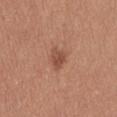Clinical impression: The lesion was tiled from a total-body skin photograph and was not biopsied. Background: Located on the lower back. Measured at roughly 2.5 mm in maximum diameter. The total-body-photography lesion software estimated a footprint of about 3.5 mm², an eccentricity of roughly 0.65, and a symmetry-axis asymmetry near 0.35. It also reported roughly 10 lightness units darker than nearby skin and a normalized lesion–skin contrast near 7. The analysis additionally found a border-irregularity index near 3/10 and a within-lesion color-variation index near 2/10. And it measured an automated nevus-likeness rating near 65 out of 100. Cropped from a total-body skin-imaging series; the visible field is about 15 mm. The tile uses white-light illumination. The subject is a female aged 38–42.